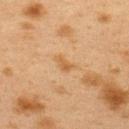notes: imaged on a skin check; not biopsied | body site: the upper back | lighting: cross-polarized | TBP lesion metrics: a lesion–skin lightness drop of about 6; a border-irregularity index near 3.5/10, internal color variation of about 1.5 on a 0–10 scale, and peripheral color asymmetry of about 0.5; a nevus-likeness score of about 0/100 and a lesion-detection confidence of about 100/100 | patient: female, about 40 years old | image: 15 mm crop, total-body photography | diameter: about 2.5 mm.